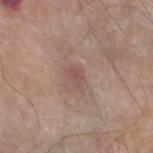Assessment:
This lesion was catalogued during total-body skin photography and was not selected for biopsy.
Context:
An algorithmic analysis of the crop reported a lesion color around L≈51 a*≈19 b*≈22 in CIELAB and a lesion-to-skin contrast of about 5.5 (normalized; higher = more distinct). The analysis additionally found an automated nevus-likeness rating near 0 out of 100. The recorded lesion diameter is about 2.5 mm. This is a white-light tile. This image is a 15 mm lesion crop taken from a total-body photograph. A male subject, aged approximately 80. The lesion is on the left thigh.